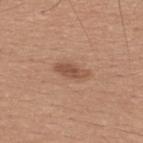Q: Is there a histopathology result?
A: catalogued during a skin exam; not biopsied
Q: Patient demographics?
A: male, approximately 30 years of age
Q: How was the tile lit?
A: white-light illumination
Q: What kind of image is this?
A: total-body-photography crop, ~15 mm field of view
Q: Where on the body is the lesion?
A: the upper back
Q: Lesion size?
A: ≈3.5 mm